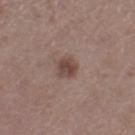Assessment:
Recorded during total-body skin imaging; not selected for excision or biopsy.
Acquisition and patient details:
A female patient, in their 50s. Automated image analysis of the tile measured an area of roughly 4.5 mm², a shape eccentricity near 0.6, and a symmetry-axis asymmetry near 0.25. The analysis additionally found a color-variation rating of about 3/10 and a peripheral color-asymmetry measure near 1. The software also gave a nevus-likeness score of about 70/100 and lesion-presence confidence of about 100/100. Measured at roughly 2.5 mm in maximum diameter. On the left thigh. Cropped from a whole-body photographic skin survey; the tile spans about 15 mm.